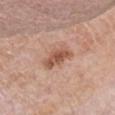Case summary:
* notes — no biopsy performed (imaged during a skin exam)
* lighting — white-light
* image source — 15 mm crop, total-body photography
* TBP lesion metrics — a nevus-likeness score of about 60/100
* location — the chest
* subject — male, aged approximately 60